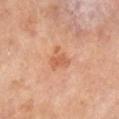follow-up: catalogued during a skin exam; not biopsied | body site: the leg | automated lesion analysis: an area of roughly 5 mm² and a symmetry-axis asymmetry near 0.6; a border-irregularity rating of about 7/10 and a peripheral color-asymmetry measure near 0.5; a detector confidence of about 100 out of 100 that the crop contains a lesion | image source: 15 mm crop, total-body photography | lesion size: ~3 mm (longest diameter) | tile lighting: cross-polarized | subject: male, aged approximately 65.